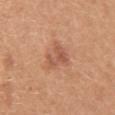Impression:
Part of a total-body skin-imaging series; this lesion was reviewed on a skin check and was not flagged for biopsy.
Image and clinical context:
Imaged with white-light lighting. A close-up tile cropped from a whole-body skin photograph, about 15 mm across. From the right upper arm. Automated image analysis of the tile measured border irregularity of about 5 on a 0–10 scale, a color-variation rating of about 3/10, and radial color variation of about 1. The software also gave a nevus-likeness score of about 50/100. The patient is a female roughly 30 years of age.